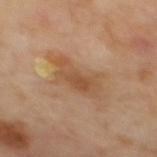workup: imaged on a skin check; not biopsied | acquisition: ~15 mm crop, total-body skin-cancer survey | size: about 5.5 mm | body site: the mid back | lighting: cross-polarized illumination | image-analysis metrics: a footprint of about 12 mm², an outline eccentricity of about 0.85 (0 = round, 1 = elongated), and two-axis asymmetry of about 0.35; border irregularity of about 5 on a 0–10 scale and radial color variation of about 1.